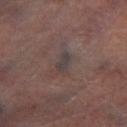Impression: Captured during whole-body skin photography for melanoma surveillance; the lesion was not biopsied. Image and clinical context: An algorithmic analysis of the crop reported a shape eccentricity near 0.85 and a symmetry-axis asymmetry near 0.3. The software also gave a border-irregularity index near 3/10, a color-variation rating of about 1/10, and a peripheral color-asymmetry measure near 0. And it measured an automated nevus-likeness rating near 0 out of 100 and lesion-presence confidence of about 80/100. The lesion is on the left lower leg. Cropped from a total-body skin-imaging series; the visible field is about 15 mm. Imaged with cross-polarized lighting. About 2.5 mm across. A male subject, approximately 65 years of age.